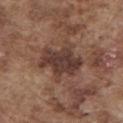Part of a total-body skin-imaging series; this lesion was reviewed on a skin check and was not flagged for biopsy.
The patient is a male aged 73–77.
The lesion-visualizer software estimated a lesion area of about 16 mm², an outline eccentricity of about 0.55 (0 = round, 1 = elongated), and two-axis asymmetry of about 0.25. It also reported border irregularity of about 4.5 on a 0–10 scale and a peripheral color-asymmetry measure near 1.5. The software also gave a detector confidence of about 95 out of 100 that the crop contains a lesion.
The lesion is on the front of the torso.
Captured under white-light illumination.
A region of skin cropped from a whole-body photographic capture, roughly 15 mm wide.
The lesion's longest dimension is about 5 mm.